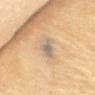Imaged during a routine full-body skin examination; the lesion was not biopsied and no histopathology is available.
From the chest.
A 15 mm close-up tile from a total-body photography series done for melanoma screening.
About 3.5 mm across.
The subject is a female aged approximately 80.